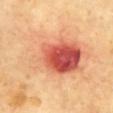Assessment:
Captured during whole-body skin photography for melanoma surveillance; the lesion was not biopsied.
Context:
A male patient aged approximately 65. This is a cross-polarized tile. The lesion's longest dimension is about 12 mm. Cropped from a whole-body photographic skin survey; the tile spans about 15 mm. The lesion is located on the chest.